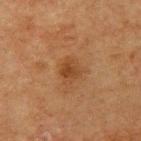Notes:
- notes · total-body-photography surveillance lesion; no biopsy
- patient · male, aged approximately 75
- imaging modality · ~15 mm crop, total-body skin-cancer survey
- anatomic site · the left upper arm